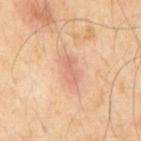Recorded during total-body skin imaging; not selected for excision or biopsy. The subject is a male approximately 55 years of age. From the mid back. This image is a 15 mm lesion crop taken from a total-body photograph. The recorded lesion diameter is about 3 mm.